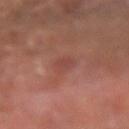Impression: No biopsy was performed on this lesion — it was imaged during a full skin examination and was not determined to be concerning. Background: The lesion is on the right forearm. A male patient, in their mid-60s. A 15 mm close-up tile from a total-body photography series done for melanoma screening. Approximately 3 mm at its widest. This is a cross-polarized tile.